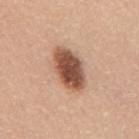follow-up = total-body-photography surveillance lesion; no biopsy
diameter = about 5.5 mm
site = the mid back
subject = male, aged 38 to 42
illumination = white-light illumination
image source = ~15 mm tile from a whole-body skin photo